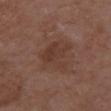Recorded during total-body skin imaging; not selected for excision or biopsy. The patient is a female approximately 80 years of age. Located on the chest. Approximately 4 mm at its widest. A lesion tile, about 15 mm wide, cut from a 3D total-body photograph.